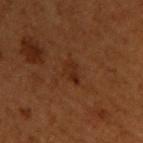follow-up = no biopsy performed (imaged during a skin exam)
image = ~15 mm crop, total-body skin-cancer survey
anatomic site = the front of the torso
subject = male, in their 50s